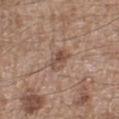{
  "biopsy_status": "not biopsied; imaged during a skin examination",
  "image": {
    "source": "total-body photography crop",
    "field_of_view_mm": 15
  },
  "automated_metrics": {
    "area_mm2_approx": 4.0,
    "eccentricity": 0.8,
    "shape_asymmetry": 0.25,
    "vs_skin_darker_L": 9.0,
    "vs_skin_contrast_norm": 7.0,
    "border_irregularity_0_10": 2.5,
    "color_variation_0_10": 5.0,
    "nevus_likeness_0_100": 5,
    "lesion_detection_confidence_0_100": 100
  },
  "lesion_size": {
    "long_diameter_mm_approx": 3.0
  },
  "site": "leg",
  "patient": {
    "sex": "male",
    "age_approx": 65
  },
  "lighting": "white-light"
}